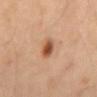Captured during whole-body skin photography for melanoma surveillance; the lesion was not biopsied.
The tile uses cross-polarized illumination.
The lesion's longest dimension is about 3 mm.
Automated image analysis of the tile measured a border-irregularity index near 2/10, internal color variation of about 3.5 on a 0–10 scale, and a peripheral color-asymmetry measure near 1. And it measured a nevus-likeness score of about 100/100 and a detector confidence of about 100 out of 100 that the crop contains a lesion.
The lesion is located on the mid back.
A male subject approximately 45 years of age.
This image is a 15 mm lesion crop taken from a total-body photograph.